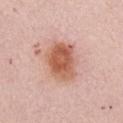Recorded during total-body skin imaging; not selected for excision or biopsy. A female subject about 65 years old. A close-up tile cropped from a whole-body skin photograph, about 15 mm across. The lesion is located on the front of the torso. The recorded lesion diameter is about 5 mm. Automated tile analysis of the lesion measured an area of roughly 15 mm² and a shape-asymmetry score of about 0.15 (0 = symmetric). And it measured a border-irregularity rating of about 2/10, internal color variation of about 5.5 on a 0–10 scale, and peripheral color asymmetry of about 1.5. The analysis additionally found a classifier nevus-likeness of about 100/100 and a lesion-detection confidence of about 100/100.